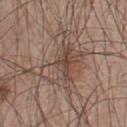illumination=white-light | subject=male, aged 48–52 | lesion size=about 6.5 mm | site=the upper back | image source=15 mm crop, total-body photography.